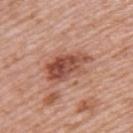biopsy status: total-body-photography surveillance lesion; no biopsy | image source: 15 mm crop, total-body photography | patient: female, aged 48–52 | body site: the upper back.